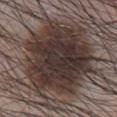The lesion was photographed on a routine skin check and not biopsied; there is no pathology result. Located on the chest. A male patient about 65 years old. A 15 mm close-up extracted from a 3D total-body photography capture.